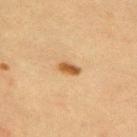Automated tile analysis of the lesion measured an average lesion color of about L≈48 a*≈19 b*≈36 (CIELAB), roughly 12 lightness units darker than nearby skin, and a lesion-to-skin contrast of about 9.5 (normalized; higher = more distinct). And it measured a detector confidence of about 100 out of 100 that the crop contains a lesion.
A male patient about 60 years old.
Longest diameter approximately 2.5 mm.
A 15 mm close-up extracted from a 3D total-body photography capture.
Imaged with cross-polarized lighting.
The lesion is located on the back.